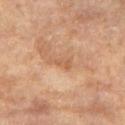Notes:
• subject — female, about 70 years old
• location — the leg
• image — ~15 mm crop, total-body skin-cancer survey